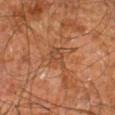Assessment: Imaged during a routine full-body skin examination; the lesion was not biopsied and no histopathology is available. Clinical summary: The lesion's longest dimension is about 3 mm. Captured under cross-polarized illumination. On the right leg. A male patient, aged 58–62. A 15 mm crop from a total-body photograph taken for skin-cancer surveillance.